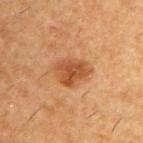biopsy status=catalogued during a skin exam; not biopsied | illumination=cross-polarized illumination | location=the right upper arm | acquisition=total-body-photography crop, ~15 mm field of view | subject=male, aged 48–52 | diameter=~4 mm (longest diameter) | TBP lesion metrics=a lesion area of about 8.5 mm² and an eccentricity of roughly 0.65; a lesion color around L≈39 a*≈21 b*≈32 in CIELAB, roughly 9 lightness units darker than nearby skin, and a normalized border contrast of about 8; a color-variation rating of about 3/10 and radial color variation of about 1; an automated nevus-likeness rating near 80 out of 100.